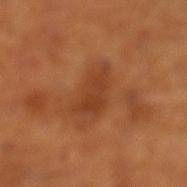Assessment: This lesion was catalogued during total-body skin photography and was not selected for biopsy. Acquisition and patient details: Automated tile analysis of the lesion measured a mean CIELAB color near L≈41 a*≈27 b*≈37, about 8 CIELAB-L* units darker than the surrounding skin, and a normalized lesion–skin contrast near 6. It also reported a border-irregularity rating of about 4/10 and a color-variation rating of about 2.5/10. This is a cross-polarized tile. The subject is a male aged 63–67. A 15 mm crop from a total-body photograph taken for skin-cancer surveillance. The lesion's longest dimension is about 4.5 mm. The lesion is on the left lower leg.